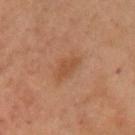notes: imaged on a skin check; not biopsied
illumination: cross-polarized
subject: female, aged 53–57
automated metrics: a lesion area of about 4 mm², an outline eccentricity of about 0.85 (0 = round, 1 = elongated), and two-axis asymmetry of about 0.25; a lesion color around L≈41 a*≈19 b*≈30 in CIELAB and roughly 6 lightness units darker than nearby skin
site: the chest
diameter: about 3 mm
image: ~15 mm crop, total-body skin-cancer survey